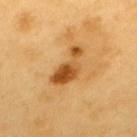The lesion was photographed on a routine skin check and not biopsied; there is no pathology result. The subject is a male about 60 years old. From the upper back. Cropped from a total-body skin-imaging series; the visible field is about 15 mm.